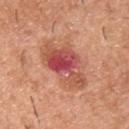Q: What is the anatomic site?
A: the upper back
Q: Lesion size?
A: about 6 mm
Q: What is the imaging modality?
A: ~15 mm crop, total-body skin-cancer survey
Q: Illumination type?
A: white-light illumination
Q: What are the patient's age and sex?
A: male, roughly 40 years of age